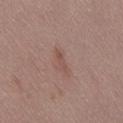The lesion was tiled from a total-body skin photograph and was not biopsied.
A 15 mm crop from a total-body photograph taken for skin-cancer surveillance.
The patient is a female aged 43 to 47.
Captured under white-light illumination.
The lesion is on the right thigh.
The lesion-visualizer software estimated an eccentricity of roughly 0.9 and a symmetry-axis asymmetry near 0.3. The analysis additionally found a mean CIELAB color near L≈51 a*≈19 b*≈24, roughly 6 lightness units darker than nearby skin, and a normalized border contrast of about 5.
Measured at roughly 2.5 mm in maximum diameter.